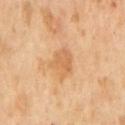{"patient": {"sex": "male", "age_approx": 65}, "image": {"source": "total-body photography crop", "field_of_view_mm": 15}}